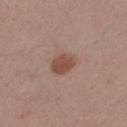The lesion was photographed on a routine skin check and not biopsied; there is no pathology result. Cropped from a whole-body photographic skin survey; the tile spans about 15 mm. A male subject, approximately 55 years of age. Measured at roughly 3 mm in maximum diameter. Located on the right upper arm. Captured under white-light illumination.